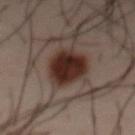Cropped from a total-body skin-imaging series; the visible field is about 15 mm.
Located on the abdomen.
A male subject aged approximately 40.